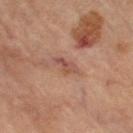Captured during whole-body skin photography for melanoma surveillance; the lesion was not biopsied. The total-body-photography lesion software estimated an outline eccentricity of about 0.9 (0 = round, 1 = elongated). The analysis additionally found a border-irregularity rating of about 4/10 and peripheral color asymmetry of about 1. From the left thigh. The tile uses cross-polarized illumination. A female patient approximately 65 years of age. Measured at roughly 3 mm in maximum diameter. A close-up tile cropped from a whole-body skin photograph, about 15 mm across.